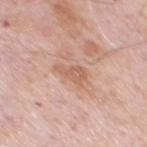Clinical impression:
Recorded during total-body skin imaging; not selected for excision or biopsy.
Clinical summary:
Located on the chest. A male subject, approximately 80 years of age. Cropped from a total-body skin-imaging series; the visible field is about 15 mm.